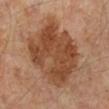Q: Was this lesion biopsied?
A: catalogued during a skin exam; not biopsied
Q: What are the patient's age and sex?
A: male, aged around 50
Q: Where on the body is the lesion?
A: the left lower leg
Q: What lighting was used for the tile?
A: cross-polarized illumination
Q: How large is the lesion?
A: ≈8 mm
Q: What kind of image is this?
A: ~15 mm tile from a whole-body skin photo